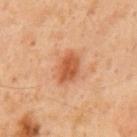The lesion was tiled from a total-body skin photograph and was not biopsied.